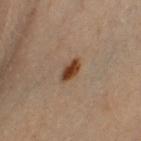This lesion was catalogued during total-body skin photography and was not selected for biopsy.